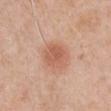{
  "biopsy_status": "not biopsied; imaged during a skin examination",
  "image": {
    "source": "total-body photography crop",
    "field_of_view_mm": 15
  },
  "lighting": "white-light",
  "automated_metrics": {
    "area_mm2_approx": 8.0,
    "eccentricity": 0.55
  },
  "lesion_size": {
    "long_diameter_mm_approx": 3.5
  },
  "site": "right upper arm",
  "patient": {
    "sex": "male",
    "age_approx": 55
  }
}